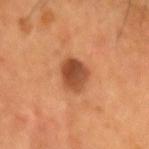| field | value |
|---|---|
| biopsy status | no biopsy performed (imaged during a skin exam) |
| image source | ~15 mm crop, total-body skin-cancer survey |
| illumination | cross-polarized |
| subject | male, in their mid- to late 50s |
| site | the head or neck |
| lesion diameter | ~3.5 mm (longest diameter) |